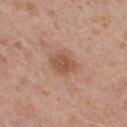Recorded during total-body skin imaging; not selected for excision or biopsy. Measured at roughly 3 mm in maximum diameter. The lesion-visualizer software estimated a border-irregularity rating of about 2/10, a color-variation rating of about 2.5/10, and a peripheral color-asymmetry measure near 0.5. The analysis additionally found an automated nevus-likeness rating near 50 out of 100 and a lesion-detection confidence of about 100/100. A 15 mm close-up extracted from a 3D total-body photography capture. A female patient, in their 40s. Located on the right thigh. Captured under white-light illumination.